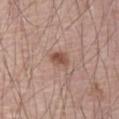Recorded during total-body skin imaging; not selected for excision or biopsy. The lesion is located on the right upper arm. Cropped from a whole-body photographic skin survey; the tile spans about 15 mm. The patient is a male aged around 70.